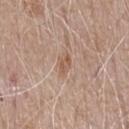<case>
  <biopsy_status>not biopsied; imaged during a skin examination</biopsy_status>
  <patient>
    <sex>male</sex>
    <age_approx>75</age_approx>
  </patient>
  <image>
    <source>total-body photography crop</source>
    <field_of_view_mm>15</field_of_view_mm>
  </image>
  <site>chest</site>
  <lighting>white-light</lighting>
  <automated_metrics>
    <eccentricity>0.85</eccentricity>
    <shape_asymmetry>0.25</shape_asymmetry>
    <vs_skin_darker_L>7.0</vs_skin_darker_L>
    <vs_skin_contrast_norm>5.5</vs_skin_contrast_norm>
    <border_irregularity_0_10>2.5</border_irregularity_0_10>
    <color_variation_0_10>2.0</color_variation_0_10>
    <peripheral_color_asymmetry>1.0</peripheral_color_asymmetry>
  </automated_metrics>
  <lesion_size>
    <long_diameter_mm_approx>3.0</long_diameter_mm_approx>
  </lesion_size>
</case>